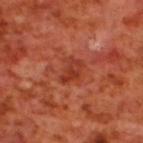Impression:
Imaged during a routine full-body skin examination; the lesion was not biopsied and no histopathology is available.
Acquisition and patient details:
A roughly 15 mm field-of-view crop from a total-body skin photograph. This is a cross-polarized tile. The subject is a male aged around 70. About 3 mm across. The lesion-visualizer software estimated a lesion area of about 5.5 mm², an eccentricity of roughly 0.75, and two-axis asymmetry of about 0.35. The analysis additionally found a border-irregularity rating of about 3.5/10 and a peripheral color-asymmetry measure near 1. It also reported a classifier nevus-likeness of about 0/100 and a lesion-detection confidence of about 100/100. On the upper back.